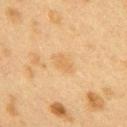The lesion was photographed on a routine skin check and not biopsied; there is no pathology result.